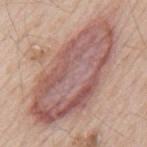The lesion was tiled from a total-body skin photograph and was not biopsied.
A male patient, in their mid- to late 60s.
A lesion tile, about 15 mm wide, cut from a 3D total-body photograph.
The lesion-visualizer software estimated a border-irregularity index near 3.5/10, internal color variation of about 8 on a 0–10 scale, and a peripheral color-asymmetry measure near 2.5.
On the mid back.
The tile uses white-light illumination.
The lesion's longest dimension is about 14 mm.